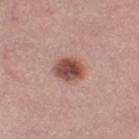biopsy status: catalogued during a skin exam; not biopsied
patient: female, aged 33 to 37
image source: total-body-photography crop, ~15 mm field of view
body site: the left thigh
TBP lesion metrics: a lesion area of about 8 mm², a shape eccentricity near 0.7, and a symmetry-axis asymmetry near 0.1; a nevus-likeness score of about 95/100 and lesion-presence confidence of about 100/100
size: ≈3.5 mm
illumination: white-light illumination